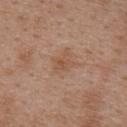| key | value |
|---|---|
| notes | no biopsy performed (imaged during a skin exam) |